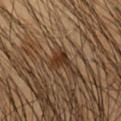Impression:
Part of a total-body skin-imaging series; this lesion was reviewed on a skin check and was not flagged for biopsy.
Image and clinical context:
This is a cross-polarized tile. A male patient approximately 45 years of age. A 15 mm close-up extracted from a 3D total-body photography capture. The lesion is located on the arm. The total-body-photography lesion software estimated a footprint of about 4 mm² and a shape-asymmetry score of about 0.45 (0 = symmetric). The analysis additionally found a mean CIELAB color near L≈32 a*≈17 b*≈29, roughly 10 lightness units darker than nearby skin, and a normalized lesion–skin contrast near 9.5.